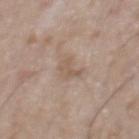Impression: Imaged during a routine full-body skin examination; the lesion was not biopsied and no histopathology is available. Context: Imaged with white-light lighting. A male patient roughly 65 years of age. Cropped from a total-body skin-imaging series; the visible field is about 15 mm. The lesion is on the chest.